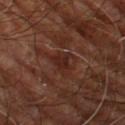Clinical impression:
No biopsy was performed on this lesion — it was imaged during a full skin examination and was not determined to be concerning.
Acquisition and patient details:
About 3 mm across. A male patient aged 58–62. A 15 mm close-up tile from a total-body photography series done for melanoma screening. The lesion is located on the right leg.